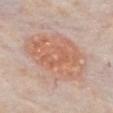| feature | finding |
|---|---|
| biopsy status | imaged on a skin check; not biopsied |
| patient | male, aged 73–77 |
| site | the chest |
| image | ~15 mm tile from a whole-body skin photo |